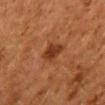Case summary:
- workup: imaged on a skin check; not biopsied
- image: ~15 mm tile from a whole-body skin photo
- subject: female, about 50 years old
- lighting: cross-polarized
- TBP lesion metrics: a lesion color around L≈30 a*≈22 b*≈30 in CIELAB, a lesion–skin lightness drop of about 9, and a normalized border contrast of about 8.5; border irregularity of about 2.5 on a 0–10 scale, a within-lesion color-variation index near 2.5/10, and peripheral color asymmetry of about 0.5; an automated nevus-likeness rating near 80 out of 100 and a lesion-detection confidence of about 100/100
- body site: the mid back